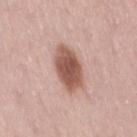Notes:
- follow-up: total-body-photography surveillance lesion; no biopsy
- TBP lesion metrics: an automated nevus-likeness rating near 100 out of 100 and lesion-presence confidence of about 100/100
- image source: ~15 mm tile from a whole-body skin photo
- size: ≈5.5 mm
- tile lighting: white-light
- site: the right thigh
- patient: female, in their 30s